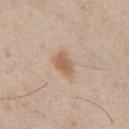No biopsy was performed on this lesion — it was imaged during a full skin examination and was not determined to be concerning. Longest diameter approximately 3.5 mm. From the chest. A male patient, aged 48 to 52. A 15 mm close-up tile from a total-body photography series done for melanoma screening.